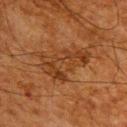No biopsy was performed on this lesion — it was imaged during a full skin examination and was not determined to be concerning. This image is a 15 mm lesion crop taken from a total-body photograph. From the upper back. The tile uses cross-polarized illumination. A male patient, roughly 65 years of age.